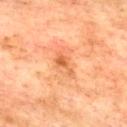This lesion was catalogued during total-body skin photography and was not selected for biopsy. The tile uses cross-polarized illumination. The lesion-visualizer software estimated a border-irregularity index near 6/10 and a within-lesion color-variation index near 1.5/10. The software also gave a nevus-likeness score of about 0/100 and lesion-presence confidence of about 100/100. A close-up tile cropped from a whole-body skin photograph, about 15 mm across. Approximately 3 mm at its widest. The lesion is on the upper back. A male patient aged 73–77.